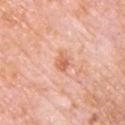biopsy status = catalogued during a skin exam; not biopsied
location = the front of the torso
subject = male, approximately 80 years of age
acquisition = ~15 mm tile from a whole-body skin photo